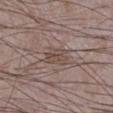biopsy_status: not biopsied; imaged during a skin examination
automated_metrics:
  border_irregularity_0_10: 3.5
  color_variation_0_10: 4.0
  peripheral_color_asymmetry: 1.5
  nevus_likeness_0_100: 0
patient:
  sex: male
  age_approx: 75
image:
  source: total-body photography crop
  field_of_view_mm: 15
site: right lower leg
lighting: white-light
lesion_size:
  long_diameter_mm_approx: 3.5